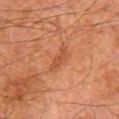- follow-up: total-body-photography surveillance lesion; no biopsy
- body site: the left thigh
- image source: ~15 mm crop, total-body skin-cancer survey
- size: about 4 mm
- subject: male, aged around 80
- automated lesion analysis: roughly 6 lightness units darker than nearby skin and a normalized lesion–skin contrast near 5; a classifier nevus-likeness of about 5/100 and a detector confidence of about 100 out of 100 that the crop contains a lesion
- tile lighting: cross-polarized illumination